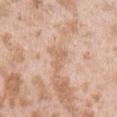Assessment: Captured during whole-body skin photography for melanoma surveillance; the lesion was not biopsied. Context: From the left upper arm. A female subject, aged approximately 25. Cropped from a total-body skin-imaging series; the visible field is about 15 mm.